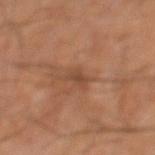Imaged during a routine full-body skin examination; the lesion was not biopsied and no histopathology is available.
The lesion is on the left lower leg.
The lesion's longest dimension is about 2.5 mm.
A 15 mm crop from a total-body photograph taken for skin-cancer surveillance.
A male patient, aged approximately 65.